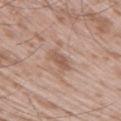The lesion was tiled from a total-body skin photograph and was not biopsied. On the arm. About 2.5 mm across. Cropped from a whole-body photographic skin survey; the tile spans about 15 mm. This is a white-light tile. The patient is a male aged 48–52.